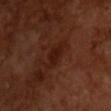Case summary:
• follow-up: no biopsy performed (imaged during a skin exam)
• TBP lesion metrics: a mean CIELAB color near L≈19 a*≈21 b*≈24, roughly 6 lightness units darker than nearby skin, and a normalized lesion–skin contrast near 7; a border-irregularity index near 3/10 and a color-variation rating of about 1/10; a nevus-likeness score of about 20/100
• image: ~15 mm crop, total-body skin-cancer survey
• tile lighting: cross-polarized
• patient: male, aged around 65
• size: ≈3.5 mm